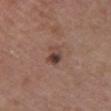Assessment:
The lesion was photographed on a routine skin check and not biopsied; there is no pathology result.
Image and clinical context:
A 15 mm crop from a total-body photograph taken for skin-cancer surveillance. About 3 mm across. Located on the chest. The tile uses white-light illumination. A female subject about 50 years old. The lesion-visualizer software estimated a peripheral color-asymmetry measure near 4.5. And it measured a detector confidence of about 100 out of 100 that the crop contains a lesion.